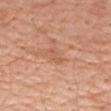Q: Was a biopsy performed?
A: total-body-photography surveillance lesion; no biopsy
Q: Lesion location?
A: the arm
Q: What kind of image is this?
A: ~15 mm tile from a whole-body skin photo
Q: What are the patient's age and sex?
A: female, approximately 65 years of age